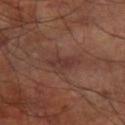Notes:
* biopsy status: imaged on a skin check; not biopsied
* automated lesion analysis: a footprint of about 7 mm², a shape eccentricity near 0.8, and a shape-asymmetry score of about 0.3 (0 = symmetric); a lesion color around L≈35 a*≈20 b*≈22 in CIELAB, a lesion–skin lightness drop of about 6, and a lesion-to-skin contrast of about 6 (normalized; higher = more distinct); a border-irregularity index near 3/10 and a within-lesion color-variation index near 3/10
* lesion size: about 4 mm
* tile lighting: cross-polarized
* acquisition: ~15 mm tile from a whole-body skin photo
* site: the right forearm
* subject: male, in their mid-60s